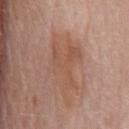{
  "biopsy_status": "not biopsied; imaged during a skin examination",
  "lesion_size": {
    "long_diameter_mm_approx": 8.0
  },
  "patient": {
    "sex": "male",
    "age_approx": 80
  },
  "image": {
    "source": "total-body photography crop",
    "field_of_view_mm": 15
  },
  "site": "chest"
}